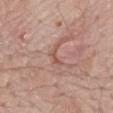workup: imaged on a skin check; not biopsied | location: the mid back | subject: male, approximately 70 years of age | automated lesion analysis: a lesion area of about 2.5 mm², an outline eccentricity of about 0.9 (0 = round, 1 = elongated), and two-axis asymmetry of about 0.6; a mean CIELAB color near L≈54 a*≈23 b*≈26 and a lesion–skin lightness drop of about 8; a border-irregularity rating of about 6.5/10, internal color variation of about 0 on a 0–10 scale, and radial color variation of about 0 | lighting: white-light | image: total-body-photography crop, ~15 mm field of view.